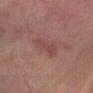Assessment:
Imaged during a routine full-body skin examination; the lesion was not biopsied and no histopathology is available.
Background:
On the right forearm. A 15 mm crop from a total-body photograph taken for skin-cancer surveillance. A male subject in their mid-60s.